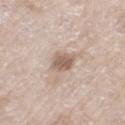biopsy status: no biopsy performed (imaged during a skin exam)
subject: female, about 75 years old
image source: ~15 mm crop, total-body skin-cancer survey
lighting: white-light illumination
location: the left thigh
lesion diameter: ≈3.5 mm